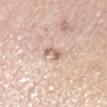This lesion was catalogued during total-body skin photography and was not selected for biopsy. A female patient aged approximately 60. Located on the left upper arm. About 2.5 mm across. A roughly 15 mm field-of-view crop from a total-body skin photograph.